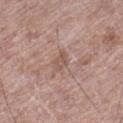Recorded during total-body skin imaging; not selected for excision or biopsy. The subject is a male about 70 years old. A 15 mm crop from a total-body photograph taken for skin-cancer surveillance. The lesion-visualizer software estimated a shape eccentricity near 0.85 and a shape-asymmetry score of about 0.25 (0 = symmetric). And it measured border irregularity of about 3 on a 0–10 scale, a within-lesion color-variation index near 1.5/10, and peripheral color asymmetry of about 0.5. It also reported a nevus-likeness score of about 0/100 and a lesion-detection confidence of about 95/100. This is a white-light tile. The recorded lesion diameter is about 2.5 mm. The lesion is on the left thigh.